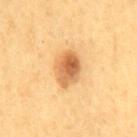Clinical impression: No biopsy was performed on this lesion — it was imaged during a full skin examination and was not determined to be concerning.